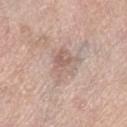Imaged during a routine full-body skin examination; the lesion was not biopsied and no histopathology is available.
A roughly 15 mm field-of-view crop from a total-body skin photograph.
Automated tile analysis of the lesion measured an eccentricity of roughly 0.35 and two-axis asymmetry of about 0.35. And it measured an average lesion color of about L≈61 a*≈17 b*≈24 (CIELAB), a lesion–skin lightness drop of about 8, and a normalized border contrast of about 5.5. It also reported border irregularity of about 4 on a 0–10 scale, internal color variation of about 4.5 on a 0–10 scale, and a peripheral color-asymmetry measure near 1.5. And it measured an automated nevus-likeness rating near 0 out of 100 and lesion-presence confidence of about 95/100.
Measured at roughly 4 mm in maximum diameter.
The subject is a female aged 68 to 72.
Located on the right lower leg.